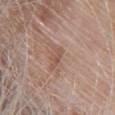Q: Is there a histopathology result?
A: imaged on a skin check; not biopsied
Q: What are the patient's age and sex?
A: female, roughly 75 years of age
Q: What did automated image analysis measure?
A: about 8 CIELAB-L* units darker than the surrounding skin and a normalized border contrast of about 5.5; an automated nevus-likeness rating near 5 out of 100 and a lesion-detection confidence of about 90/100
Q: How large is the lesion?
A: ≈3 mm
Q: What kind of image is this?
A: 15 mm crop, total-body photography
Q: How was the tile lit?
A: white-light
Q: What is the anatomic site?
A: the chest